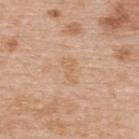This lesion was catalogued during total-body skin photography and was not selected for biopsy. The lesion is located on the upper back. The patient is a male in their 70s. A 15 mm close-up tile from a total-body photography series done for melanoma screening. This is a white-light tile. Longest diameter approximately 3 mm.